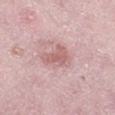Q: Is there a histopathology result?
A: imaged on a skin check; not biopsied
Q: How was this image acquired?
A: total-body-photography crop, ~15 mm field of view
Q: What are the patient's age and sex?
A: female, roughly 45 years of age
Q: Lesion location?
A: the left thigh
Q: What lighting was used for the tile?
A: white-light
Q: Automated lesion metrics?
A: an average lesion color of about L≈62 a*≈23 b*≈21 (CIELAB) and roughly 9 lightness units darker than nearby skin; border irregularity of about 4 on a 0–10 scale, a color-variation rating of about 2.5/10, and peripheral color asymmetry of about 1; a classifier nevus-likeness of about 5/100 and a detector confidence of about 100 out of 100 that the crop contains a lesion
Q: What is the lesion's diameter?
A: about 4 mm